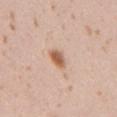The lesion was photographed on a routine skin check and not biopsied; there is no pathology result. Imaged with white-light lighting. Approximately 2.5 mm at its widest. Located on the right upper arm. An algorithmic analysis of the crop reported a lesion color around L≈60 a*≈21 b*≈32 in CIELAB, roughly 13 lightness units darker than nearby skin, and a normalized lesion–skin contrast near 9. The analysis additionally found border irregularity of about 1.5 on a 0–10 scale, a color-variation rating of about 5.5/10, and a peripheral color-asymmetry measure near 2. And it measured a detector confidence of about 100 out of 100 that the crop contains a lesion. A female subject aged 18 to 22. A 15 mm crop from a total-body photograph taken for skin-cancer surveillance.